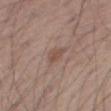Captured during whole-body skin photography for melanoma surveillance; the lesion was not biopsied. From the right thigh. A male patient aged 53 to 57. The lesion's longest dimension is about 2.5 mm. A roughly 15 mm field-of-view crop from a total-body skin photograph.